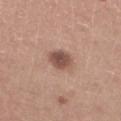Q: Is there a histopathology result?
A: no biopsy performed (imaged during a skin exam)
Q: How was the tile lit?
A: white-light illumination
Q: What is the imaging modality?
A: ~15 mm tile from a whole-body skin photo
Q: What are the patient's age and sex?
A: male, aged approximately 60
Q: Lesion location?
A: the left lower leg
Q: What did automated image analysis measure?
A: a lesion area of about 6.5 mm² and two-axis asymmetry of about 0.15